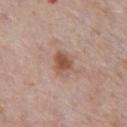| key | value |
|---|---|
| biopsy status | catalogued during a skin exam; not biopsied |
| TBP lesion metrics | a lesion area of about 6.5 mm² and a symmetry-axis asymmetry near 0.15; a border-irregularity rating of about 1.5/10, a color-variation rating of about 4.5/10, and radial color variation of about 1.5; a classifier nevus-likeness of about 80/100 and a lesion-detection confidence of about 100/100 |
| image source | 15 mm crop, total-body photography |
| diameter | ~3 mm (longest diameter) |
| body site | the chest |
| patient | male, aged 68–72 |
| tile lighting | white-light illumination |